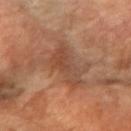The lesion is located on the arm. A region of skin cropped from a whole-body photographic capture, roughly 15 mm wide. A female patient, aged around 70. The total-body-photography lesion software estimated an area of roughly 12 mm², a shape eccentricity near 0.9, and a symmetry-axis asymmetry near 0.35. And it measured an average lesion color of about L≈45 a*≈21 b*≈30 (CIELAB) and roughly 7 lightness units darker than nearby skin. Imaged with cross-polarized lighting. The lesion's longest dimension is about 6.5 mm.